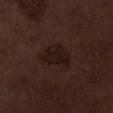workup — imaged on a skin check; not biopsied
image — ~15 mm crop, total-body skin-cancer survey
tile lighting — white-light
subject — male, aged approximately 70
lesion diameter — ~4.5 mm (longest diameter)
anatomic site — the leg